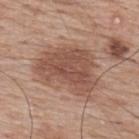{
  "biopsy_status": "not biopsied; imaged during a skin examination",
  "patient": {
    "sex": "male",
    "age_approx": 70
  },
  "site": "upper back",
  "image": {
    "source": "total-body photography crop",
    "field_of_view_mm": 15
  },
  "lighting": "white-light",
  "lesion_size": {
    "long_diameter_mm_approx": 7.5
  }
}